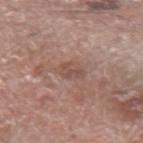Impression:
The lesion was photographed on a routine skin check and not biopsied; there is no pathology result.
Clinical summary:
Automated tile analysis of the lesion measured a lesion area of about 5 mm² and an eccentricity of roughly 0.85. And it measured a lesion color around L≈51 a*≈19 b*≈24 in CIELAB, about 7 CIELAB-L* units darker than the surrounding skin, and a normalized lesion–skin contrast near 5.5. It also reported a classifier nevus-likeness of about 0/100 and lesion-presence confidence of about 100/100. Cropped from a total-body skin-imaging series; the visible field is about 15 mm. The tile uses white-light illumination. The lesion's longest dimension is about 3.5 mm. A male subject aged 58–62. The lesion is on the arm.